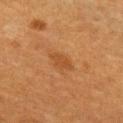follow-up: total-body-photography surveillance lesion; no biopsy | body site: the chest | subject: female, in their mid- to late 50s | illumination: cross-polarized illumination | automated metrics: a lesion area of about 3.5 mm² and two-axis asymmetry of about 0.25; an average lesion color of about L≈41 a*≈22 b*≈36 (CIELAB), about 6 CIELAB-L* units darker than the surrounding skin, and a normalized border contrast of about 6; border irregularity of about 2.5 on a 0–10 scale, a color-variation rating of about 1/10, and radial color variation of about 0.5 | image: 15 mm crop, total-body photography.